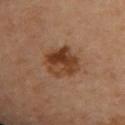Recorded during total-body skin imaging; not selected for excision or biopsy. The subject is a female aged 43–47. Located on the back. The tile uses cross-polarized illumination. A close-up tile cropped from a whole-body skin photograph, about 15 mm across. An algorithmic analysis of the crop reported an average lesion color of about L≈41 a*≈22 b*≈33 (CIELAB), a lesion–skin lightness drop of about 12, and a lesion-to-skin contrast of about 9.5 (normalized; higher = more distinct). The analysis additionally found radial color variation of about 3.5. And it measured a classifier nevus-likeness of about 85/100. About 4.5 mm across.